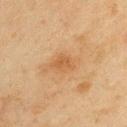Part of a total-body skin-imaging series; this lesion was reviewed on a skin check and was not flagged for biopsy. The subject is a male aged approximately 45. Captured under cross-polarized illumination. About 3 mm across. Automated tile analysis of the lesion measured an average lesion color of about L≈47 a*≈18 b*≈35 (CIELAB), about 6 CIELAB-L* units darker than the surrounding skin, and a normalized border contrast of about 6. Located on the arm. A close-up tile cropped from a whole-body skin photograph, about 15 mm across.